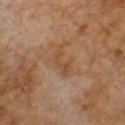Clinical impression: This lesion was catalogued during total-body skin photography and was not selected for biopsy. Image and clinical context: A male subject, aged around 80. The recorded lesion diameter is about 4 mm. A 15 mm close-up tile from a total-body photography series done for melanoma screening. The lesion is on the chest. The tile uses cross-polarized illumination. Automated image analysis of the tile measured a mean CIELAB color near L≈36 a*≈15 b*≈26, about 5 CIELAB-L* units darker than the surrounding skin, and a lesion-to-skin contrast of about 5.5 (normalized; higher = more distinct).